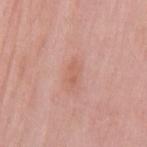No biopsy was performed on this lesion — it was imaged during a full skin examination and was not determined to be concerning. Captured under white-light illumination. A 15 mm crop from a total-body photograph taken for skin-cancer surveillance. The recorded lesion diameter is about 2.5 mm. A female subject aged 58 to 62. The lesion is located on the mid back.